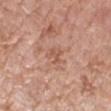TBP lesion metrics=a border-irregularity index near 5.5/10, internal color variation of about 2 on a 0–10 scale, and peripheral color asymmetry of about 1 | subject=female, aged approximately 70 | lesion diameter=about 3 mm | body site=the right forearm | image source=~15 mm tile from a whole-body skin photo | lighting=white-light illumination.